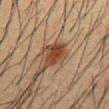– follow-up — total-body-photography surveillance lesion; no biopsy
– illumination — cross-polarized
– subject — male, approximately 35 years of age
– body site — the abdomen
– automated lesion analysis — an area of roughly 6 mm², an outline eccentricity of about 0.65 (0 = round, 1 = elongated), and two-axis asymmetry of about 0.3; a nevus-likeness score of about 95/100 and a detector confidence of about 100 out of 100 that the crop contains a lesion
– image source — 15 mm crop, total-body photography
– diameter — ~3 mm (longest diameter)